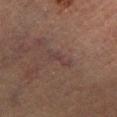The lesion was photographed on a routine skin check and not biopsied; there is no pathology result. Longest diameter approximately 3 mm. The total-body-photography lesion software estimated a shape eccentricity near 0.9 and a shape-asymmetry score of about 0.35 (0 = symmetric). The software also gave an average lesion color of about L≈33 a*≈15 b*≈17 (CIELAB), about 4 CIELAB-L* units darker than the surrounding skin, and a normalized border contrast of about 5. The software also gave a border-irregularity rating of about 5/10, internal color variation of about 0.5 on a 0–10 scale, and a peripheral color-asymmetry measure near 0. The lesion is located on the left lower leg. Cropped from a total-body skin-imaging series; the visible field is about 15 mm. A male subject about 85 years old or older. Captured under cross-polarized illumination.